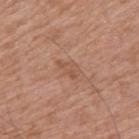Clinical impression: The lesion was tiled from a total-body skin photograph and was not biopsied. Acquisition and patient details: The lesion is located on the right upper arm. Automated image analysis of the tile measured a footprint of about 3.5 mm², a shape eccentricity near 0.85, and two-axis asymmetry of about 0.45. The analysis additionally found a lesion color around L≈53 a*≈22 b*≈31 in CIELAB, about 6 CIELAB-L* units darker than the surrounding skin, and a normalized border contrast of about 5. And it measured internal color variation of about 1.5 on a 0–10 scale and a peripheral color-asymmetry measure near 0.5. A male patient, aged approximately 60. Captured under white-light illumination. A 15 mm close-up tile from a total-body photography series done for melanoma screening.